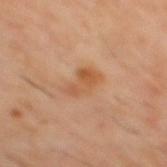biopsy status: imaged on a skin check; not biopsied | lighting: cross-polarized | patient: male, in their 60s | TBP lesion metrics: a footprint of about 7 mm², a shape eccentricity near 0.85, and two-axis asymmetry of about 0.3; border irregularity of about 3.5 on a 0–10 scale and internal color variation of about 4.5 on a 0–10 scale | lesion size: ≈4 mm | anatomic site: the mid back | acquisition: total-body-photography crop, ~15 mm field of view.